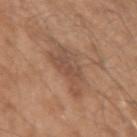Captured during whole-body skin photography for melanoma surveillance; the lesion was not biopsied. A 15 mm close-up extracted from a 3D total-body photography capture. The subject is a male roughly 50 years of age. About 5 mm across. The lesion is located on the left upper arm. Captured under white-light illumination. Automated image analysis of the tile measured internal color variation of about 4 on a 0–10 scale and peripheral color asymmetry of about 1.5. The analysis additionally found a classifier nevus-likeness of about 5/100 and a detector confidence of about 100 out of 100 that the crop contains a lesion.